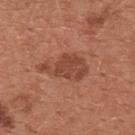Clinical impression:
The lesion was photographed on a routine skin check and not biopsied; there is no pathology result.
Clinical summary:
This image is a 15 mm lesion crop taken from a total-body photograph. Imaged with white-light lighting. Longest diameter approximately 5.5 mm. A female patient, in their mid-30s. The lesion is on the chest.